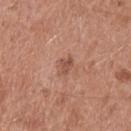Captured during whole-body skin photography for melanoma surveillance; the lesion was not biopsied. The recorded lesion diameter is about 2.5 mm. A 15 mm crop from a total-body photograph taken for skin-cancer surveillance. A male patient, aged approximately 55. Imaged with white-light lighting. The lesion is on the chest. Automated tile analysis of the lesion measured a footprint of about 3.5 mm², a shape eccentricity near 0.75, and two-axis asymmetry of about 0.25. And it measured a nevus-likeness score of about 20/100 and lesion-presence confidence of about 100/100.